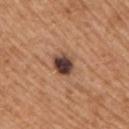notes = no biopsy performed (imaged during a skin exam) | subject = male, roughly 65 years of age | anatomic site = the right upper arm | lighting = white-light | image source = ~15 mm crop, total-body skin-cancer survey.